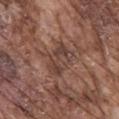The lesion was photographed on a routine skin check and not biopsied; there is no pathology result. On the mid back. The lesion's longest dimension is about 4 mm. Automated tile analysis of the lesion measured an average lesion color of about L≈41 a*≈19 b*≈23 (CIELAB), about 8 CIELAB-L* units darker than the surrounding skin, and a lesion-to-skin contrast of about 6.5 (normalized; higher = more distinct). The software also gave a nevus-likeness score of about 0/100 and a detector confidence of about 75 out of 100 that the crop contains a lesion. The subject is a male aged 73–77. Cropped from a total-body skin-imaging series; the visible field is about 15 mm.